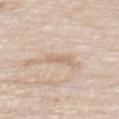Clinical impression: Recorded during total-body skin imaging; not selected for excision or biopsy. Image and clinical context: Imaged with white-light lighting. A male subject about 85 years old or older. The lesion is on the upper back. A 15 mm crop from a total-body photograph taken for skin-cancer surveillance. The total-body-photography lesion software estimated a peripheral color-asymmetry measure near 0. It also reported a classifier nevus-likeness of about 0/100.